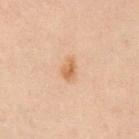Clinical impression:
The lesion was photographed on a routine skin check and not biopsied; there is no pathology result.
Acquisition and patient details:
A female subject, approximately 30 years of age. The lesion is located on the left upper arm. A close-up tile cropped from a whole-body skin photograph, about 15 mm across. Approximately 2.5 mm at its widest.